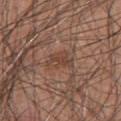biopsy status: catalogued during a skin exam; not biopsied | subject: male, aged 58 to 62 | site: the chest | automated metrics: a footprint of about 4.5 mm² and an outline eccentricity of about 0.85 (0 = round, 1 = elongated); a mean CIELAB color near L≈43 a*≈20 b*≈26 and a normalized border contrast of about 6; a border-irregularity index near 4.5/10, internal color variation of about 1.5 on a 0–10 scale, and a peripheral color-asymmetry measure near 0.5 | lesion diameter: about 3.5 mm | imaging modality: 15 mm crop, total-body photography.